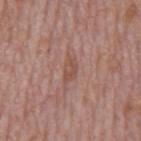The lesion was photographed on a routine skin check and not biopsied; there is no pathology result.
A male patient, aged around 75.
The recorded lesion diameter is about 3 mm.
Imaged with white-light lighting.
Located on the mid back.
A 15 mm close-up tile from a total-body photography series done for melanoma screening.
The lesion-visualizer software estimated an eccentricity of roughly 0.85 and a symmetry-axis asymmetry near 0.35. And it measured a lesion color around L≈50 a*≈22 b*≈26 in CIELAB, about 7 CIELAB-L* units darker than the surrounding skin, and a lesion-to-skin contrast of about 5.5 (normalized; higher = more distinct). The analysis additionally found a border-irregularity rating of about 3.5/10, internal color variation of about 1.5 on a 0–10 scale, and radial color variation of about 0.5. The software also gave a lesion-detection confidence of about 100/100.